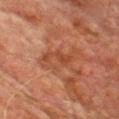<tbp_lesion>
  <automated_metrics>
    <shape_asymmetry>0.35</shape_asymmetry>
    <vs_skin_darker_L>6.0</vs_skin_darker_L>
    <vs_skin_contrast_norm>5.5</vs_skin_contrast_norm>
    <nevus_likeness_0_100>0</nevus_likeness_0_100>
    <lesion_detection_confidence_0_100>100</lesion_detection_confidence_0_100>
  </automated_metrics>
  <lighting>cross-polarized</lighting>
  <patient>
    <sex>male</sex>
    <age_approx>75</age_approx>
  </patient>
  <site>chest</site>
  <lesion_size>
    <long_diameter_mm_approx>5.5</long_diameter_mm_approx>
  </lesion_size>
  <image>
    <source>total-body photography crop</source>
    <field_of_view_mm>15</field_of_view_mm>
  </image>
</tbp_lesion>